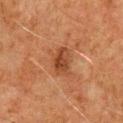No biopsy was performed on this lesion — it was imaged during a full skin examination and was not determined to be concerning. A male patient, aged around 80. The lesion is located on the chest. The total-body-photography lesion software estimated a lesion area of about 7 mm², an eccentricity of roughly 0.75, and a shape-asymmetry score of about 0.25 (0 = symmetric). And it measured a border-irregularity index near 2/10 and peripheral color asymmetry of about 1.5. And it measured a nevus-likeness score of about 40/100 and a lesion-detection confidence of about 100/100. Cropped from a total-body skin-imaging series; the visible field is about 15 mm. This is a cross-polarized tile.